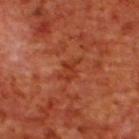Assessment: The lesion was tiled from a total-body skin photograph and was not biopsied. Clinical summary: A male subject aged 68–72. Longest diameter approximately 3 mm. The total-body-photography lesion software estimated a lesion color around L≈37 a*≈32 b*≈36 in CIELAB, roughly 6 lightness units darker than nearby skin, and a normalized border contrast of about 6. And it measured a classifier nevus-likeness of about 0/100 and lesion-presence confidence of about 100/100. Located on the upper back. Cropped from a whole-body photographic skin survey; the tile spans about 15 mm.